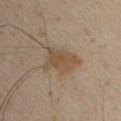Automated image analysis of the tile measured an average lesion color of about L≈43 a*≈12 b*≈27 (CIELAB), a lesion–skin lightness drop of about 8, and a normalized border contrast of about 7. It also reported border irregularity of about 4 on a 0–10 scale, internal color variation of about 2.5 on a 0–10 scale, and a peripheral color-asymmetry measure near 1. And it measured a detector confidence of about 100 out of 100 that the crop contains a lesion.
A close-up tile cropped from a whole-body skin photograph, about 15 mm across.
The subject is a male approximately 50 years of age.
Longest diameter approximately 4.5 mm.
On the chest.